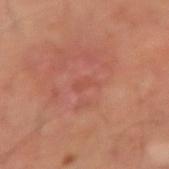Case summary:
• biopsy status — no biopsy performed (imaged during a skin exam)
• image — total-body-photography crop, ~15 mm field of view
• automated metrics — a lesion area of about 2.5 mm², a shape eccentricity near 0.85, and a shape-asymmetry score of about 0.35 (0 = symmetric); an average lesion color of about L≈50 a*≈29 b*≈29 (CIELAB) and a normalized lesion–skin contrast near 3.5; an automated nevus-likeness rating near 0 out of 100 and lesion-presence confidence of about 100/100
• illumination — cross-polarized
• site — the left forearm
• subject — male, in their mid- to late 40s
• lesion diameter — about 2.5 mm